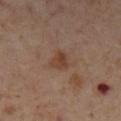  site: left lower leg
  lesion_size:
    long_diameter_mm_approx: 2.5
  patient:
    sex: female
    age_approx: 55
  image:
    source: total-body photography crop
    field_of_view_mm: 15